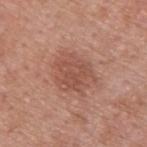<lesion>
<biopsy_status>not biopsied; imaged during a skin examination</biopsy_status>
<lesion_size>
  <long_diameter_mm_approx>5.0</long_diameter_mm_approx>
</lesion_size>
<lighting>white-light</lighting>
<site>upper back</site>
<patient>
  <sex>male</sex>
  <age_approx>55</age_approx>
</patient>
<image>
  <source>total-body photography crop</source>
  <field_of_view_mm>15</field_of_view_mm>
</image>
</lesion>